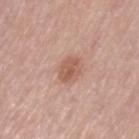Assessment: Imaged during a routine full-body skin examination; the lesion was not biopsied and no histopathology is available. Context: The subject is a female about 65 years old. The lesion is on the right thigh. Automated image analysis of the tile measured a shape eccentricity near 0.7 and two-axis asymmetry of about 0.25. Longest diameter approximately 3 mm. A roughly 15 mm field-of-view crop from a total-body skin photograph. The tile uses white-light illumination.